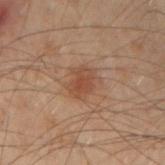{"biopsy_status": "not biopsied; imaged during a skin examination", "patient": {"sex": "male", "age_approx": 50}, "lighting": "cross-polarized", "automated_metrics": {"cielab_L": 38, "cielab_a": 18, "cielab_b": 26, "vs_skin_darker_L": 7.0, "vs_skin_contrast_norm": 6.5, "nevus_likeness_0_100": 60, "lesion_detection_confidence_0_100": 100}, "image": {"source": "total-body photography crop", "field_of_view_mm": 15}, "site": "left forearm", "lesion_size": {"long_diameter_mm_approx": 3.0}}